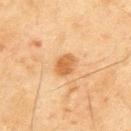image:
  source: total-body photography crop
  field_of_view_mm: 15
lighting: cross-polarized
site: upper back
patient:
  sex: male
  age_approx: 45